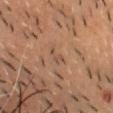The lesion was tiled from a total-body skin photograph and was not biopsied. The recorded lesion diameter is about 2.5 mm. A male patient aged 43–47. This is a cross-polarized tile. Cropped from a total-body skin-imaging series; the visible field is about 15 mm. Automated image analysis of the tile measured an average lesion color of about L≈47 a*≈17 b*≈27 (CIELAB), roughly 6 lightness units darker than nearby skin, and a normalized lesion–skin contrast near 5. The analysis additionally found a nevus-likeness score of about 0/100 and a lesion-detection confidence of about 80/100. Located on the chest.